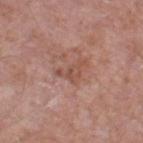Image and clinical context: The total-body-photography lesion software estimated a footprint of about 4.5 mm², a shape eccentricity near 0.85, and a symmetry-axis asymmetry near 0.65. And it measured a normalized lesion–skin contrast near 6. It also reported a within-lesion color-variation index near 2.5/10 and peripheral color asymmetry of about 1. The software also gave an automated nevus-likeness rating near 0 out of 100. From the leg. A roughly 15 mm field-of-view crop from a total-body skin photograph. The recorded lesion diameter is about 4 mm. A male patient aged 58–62.